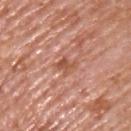biopsy status: catalogued during a skin exam; not biopsied | image source: ~15 mm tile from a whole-body skin photo | patient: male, aged 73 to 77 | diameter: ≈2.5 mm | location: the chest | lighting: white-light.